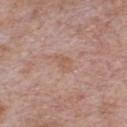imaging modality — 15 mm crop, total-body photography | subject — male, aged around 60 | diameter — about 2.5 mm | body site — the chest | image-analysis metrics — an outline eccentricity of about 0.75 (0 = round, 1 = elongated) and a symmetry-axis asymmetry near 0.25; roughly 6 lightness units darker than nearby skin and a normalized lesion–skin contrast near 5.5; a nevus-likeness score of about 0/100 and lesion-presence confidence of about 100/100.